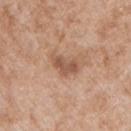Impression: This lesion was catalogued during total-body skin photography and was not selected for biopsy. Background: The subject is a male roughly 65 years of age. Captured under white-light illumination. An algorithmic analysis of the crop reported a mean CIELAB color near L≈54 a*≈21 b*≈30, about 11 CIELAB-L* units darker than the surrounding skin, and a lesion-to-skin contrast of about 7.5 (normalized; higher = more distinct). And it measured a classifier nevus-likeness of about 15/100 and lesion-presence confidence of about 100/100. The lesion is on the chest. The lesion's longest dimension is about 3 mm. Cropped from a total-body skin-imaging series; the visible field is about 15 mm.